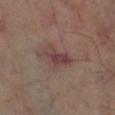Recorded during total-body skin imaging; not selected for excision or biopsy.
Cropped from a total-body skin-imaging series; the visible field is about 15 mm.
This is a cross-polarized tile.
From the leg.
An algorithmic analysis of the crop reported an average lesion color of about L≈39 a*≈19 b*≈18 (CIELAB), about 8 CIELAB-L* units darker than the surrounding skin, and a lesion-to-skin contrast of about 7.5 (normalized; higher = more distinct). The software also gave a classifier nevus-likeness of about 5/100 and lesion-presence confidence of about 100/100.
A male patient, aged 68–72.
The recorded lesion diameter is about 4 mm.